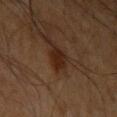Impression:
No biopsy was performed on this lesion — it was imaged during a full skin examination and was not determined to be concerning.
Background:
The lesion's longest dimension is about 3 mm. An algorithmic analysis of the crop reported an average lesion color of about L≈21 a*≈16 b*≈23 (CIELAB), about 7 CIELAB-L* units darker than the surrounding skin, and a normalized border contrast of about 9. The software also gave border irregularity of about 3 on a 0–10 scale, a within-lesion color-variation index near 2/10, and peripheral color asymmetry of about 1. The analysis additionally found a classifier nevus-likeness of about 85/100 and a lesion-detection confidence of about 100/100. The lesion is on the right upper arm. The subject is a male roughly 60 years of age. This is a cross-polarized tile. A 15 mm close-up tile from a total-body photography series done for melanoma screening.